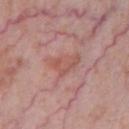Clinical impression: Part of a total-body skin-imaging series; this lesion was reviewed on a skin check and was not flagged for biopsy. Clinical summary: Located on the chest. The subject is a male approximately 60 years of age. About 4.5 mm across. This is a white-light tile. A lesion tile, about 15 mm wide, cut from a 3D total-body photograph. The total-body-photography lesion software estimated a footprint of about 8.5 mm², an outline eccentricity of about 0.75 (0 = round, 1 = elongated), and two-axis asymmetry of about 0.4. The software also gave a lesion color around L≈55 a*≈24 b*≈25 in CIELAB, about 7 CIELAB-L* units darker than the surrounding skin, and a normalized border contrast of about 5.5. It also reported a within-lesion color-variation index near 3.5/10 and radial color variation of about 1. The analysis additionally found a nevus-likeness score of about 0/100 and a detector confidence of about 90 out of 100 that the crop contains a lesion.